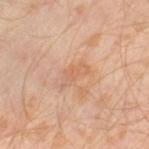| field | value |
|---|---|
| notes | imaged on a skin check; not biopsied |
| lighting | cross-polarized illumination |
| image-analysis metrics | an area of roughly 4 mm²; a border-irregularity rating of about 4/10, a within-lesion color-variation index near 1/10, and a peripheral color-asymmetry measure near 0.5; an automated nevus-likeness rating near 0 out of 100 |
| patient | male, aged approximately 30 |
| acquisition | total-body-photography crop, ~15 mm field of view |
| anatomic site | the leg |
| lesion size | ≈3.5 mm |